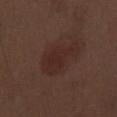Clinical impression:
Part of a total-body skin-imaging series; this lesion was reviewed on a skin check and was not flagged for biopsy.
Acquisition and patient details:
From the left thigh. The patient is a male aged 68–72. Imaged with white-light lighting. Cropped from a total-body skin-imaging series; the visible field is about 15 mm. About 6 mm across.